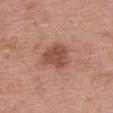Recorded during total-body skin imaging; not selected for excision or biopsy. The subject is a female aged 63 to 67. The lesion's longest dimension is about 4 mm. A roughly 15 mm field-of-view crop from a total-body skin photograph. The lesion is located on the upper back.